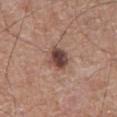Recorded during total-body skin imaging; not selected for excision or biopsy. A male subject aged 73 to 77. Automated tile analysis of the lesion measured radial color variation of about 2. The lesion is on the abdomen. A 15 mm close-up tile from a total-body photography series done for melanoma screening. This is a white-light tile.